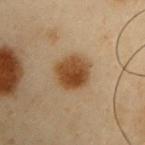No biopsy was performed on this lesion — it was imaged during a full skin examination and was not determined to be concerning.